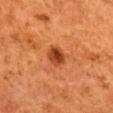Captured during whole-body skin photography for melanoma surveillance; the lesion was not biopsied. On the mid back. A female patient aged 48–52. A region of skin cropped from a whole-body photographic capture, roughly 15 mm wide.